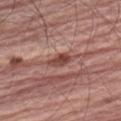biopsy status — total-body-photography surveillance lesion; no biopsy | illumination — white-light | location — the left upper arm | subject — male, aged 63 to 67 | lesion size — ~3 mm (longest diameter) | automated lesion analysis — a lesion area of about 4 mm² and a symmetry-axis asymmetry near 0.3; a mean CIELAB color near L≈45 a*≈25 b*≈25, a lesion–skin lightness drop of about 12, and a normalized border contrast of about 9; a classifier nevus-likeness of about 10/100 and a lesion-detection confidence of about 90/100 | image — ~15 mm crop, total-body skin-cancer survey.